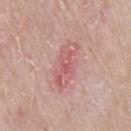workup — total-body-photography surveillance lesion; no biopsy | lesion size — ≈6 mm | anatomic site — the abdomen | automated metrics — a footprint of about 12 mm² and an outline eccentricity of about 0.9 (0 = round, 1 = elongated); a border-irregularity rating of about 3.5/10, a within-lesion color-variation index near 5.5/10, and peripheral color asymmetry of about 2; a classifier nevus-likeness of about 0/100 and lesion-presence confidence of about 100/100 | imaging modality — ~15 mm crop, total-body skin-cancer survey | lighting — white-light illumination | patient — male, aged around 65.